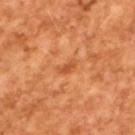{"image": {"source": "total-body photography crop", "field_of_view_mm": 15}, "patient": {"sex": "male", "age_approx": 65}}